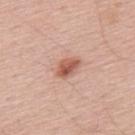No biopsy was performed on this lesion — it was imaged during a full skin examination and was not determined to be concerning. The lesion is on the back. This is a white-light tile. A roughly 15 mm field-of-view crop from a total-body skin photograph. The patient is a male aged around 55.